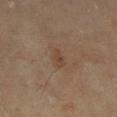Notes:
• notes: imaged on a skin check; not biopsied
• site: the left lower leg
• illumination: cross-polarized
• imaging modality: ~15 mm crop, total-body skin-cancer survey
• patient: male, approximately 65 years of age